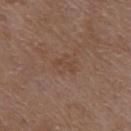About 2.5 mm across.
A female subject, aged approximately 70.
This is a white-light tile.
An algorithmic analysis of the crop reported a lesion area of about 2 mm² and an outline eccentricity of about 0.95 (0 = round, 1 = elongated). It also reported border irregularity of about 5.5 on a 0–10 scale, a within-lesion color-variation index near 0/10, and radial color variation of about 0. The software also gave a classifier nevus-likeness of about 0/100 and a lesion-detection confidence of about 100/100.
Cropped from a whole-body photographic skin survey; the tile spans about 15 mm.
Located on the upper back.